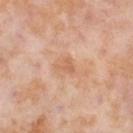| key | value |
|---|---|
| notes | catalogued during a skin exam; not biopsied |
| patient | female, in their mid- to late 50s |
| body site | the right lower leg |
| acquisition | 15 mm crop, total-body photography |